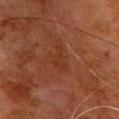- workup — total-body-photography surveillance lesion; no biopsy
- location — the chest
- subject — male, aged approximately 80
- image source — total-body-photography crop, ~15 mm field of view